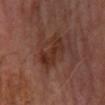workup=catalogued during a skin exam; not biopsied | acquisition=total-body-photography crop, ~15 mm field of view | lighting=cross-polarized illumination | patient=male, roughly 70 years of age | body site=the leg | lesion size=about 4 mm | automated metrics=a border-irregularity index near 5/10, a color-variation rating of about 3/10, and a peripheral color-asymmetry measure near 1; an automated nevus-likeness rating near 0 out of 100 and a lesion-detection confidence of about 100/100.